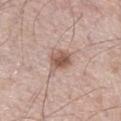No biopsy was performed on this lesion — it was imaged during a full skin examination and was not determined to be concerning.
The lesion is located on the right thigh.
A male subject, aged approximately 70.
A 15 mm close-up extracted from a 3D total-body photography capture.
The total-body-photography lesion software estimated an average lesion color of about L≈56 a*≈19 b*≈26 (CIELAB), about 12 CIELAB-L* units darker than the surrounding skin, and a normalized border contrast of about 8. And it measured a classifier nevus-likeness of about 90/100 and lesion-presence confidence of about 100/100.